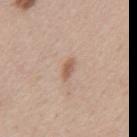{"biopsy_status": "not biopsied; imaged during a skin examination", "automated_metrics": {"border_irregularity_0_10": 2.5, "color_variation_0_10": 1.0, "peripheral_color_asymmetry": 0.5, "nevus_likeness_0_100": 80}, "site": "mid back", "lesion_size": {"long_diameter_mm_approx": 2.5}, "image": {"source": "total-body photography crop", "field_of_view_mm": 15}, "lighting": "white-light", "patient": {"sex": "male", "age_approx": 45}}